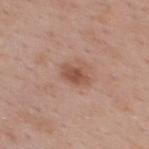The lesion's longest dimension is about 3 mm. From the upper back. A close-up tile cropped from a whole-body skin photograph, about 15 mm across. A female patient aged 33–37.